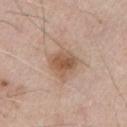Imaged during a routine full-body skin examination; the lesion was not biopsied and no histopathology is available.
Approximately 3.5 mm at its widest.
The tile uses white-light illumination.
The subject is a male aged 68 to 72.
A 15 mm crop from a total-body photograph taken for skin-cancer surveillance.
From the chest.
Automated image analysis of the tile measured an eccentricity of roughly 0.35 and two-axis asymmetry of about 0.25. The software also gave a mean CIELAB color near L≈56 a*≈18 b*≈30, roughly 11 lightness units darker than nearby skin, and a normalized lesion–skin contrast near 7.5. The analysis additionally found a border-irregularity rating of about 2.5/10, a color-variation rating of about 4.5/10, and peripheral color asymmetry of about 1.5. The analysis additionally found an automated nevus-likeness rating near 75 out of 100 and a lesion-detection confidence of about 100/100.